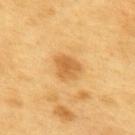Q: Was this lesion biopsied?
A: no biopsy performed (imaged during a skin exam)
Q: Lesion location?
A: the upper back
Q: Lesion size?
A: ~3 mm (longest diameter)
Q: Who is the patient?
A: male, aged 58–62
Q: Illumination type?
A: cross-polarized
Q: How was this image acquired?
A: total-body-photography crop, ~15 mm field of view